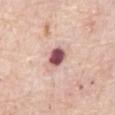Case summary:
– follow-up — imaged on a skin check; not biopsied
– subject — male, in their 80s
– illumination — white-light
– image — total-body-photography crop, ~15 mm field of view
– anatomic site — the abdomen